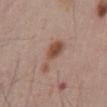Assessment:
Captured during whole-body skin photography for melanoma surveillance; the lesion was not biopsied.
Acquisition and patient details:
Automated image analysis of the tile measured a lesion-detection confidence of about 100/100. The recorded lesion diameter is about 5 mm. Captured under white-light illumination. The patient is a male aged 53–57. Cropped from a whole-body photographic skin survey; the tile spans about 15 mm. From the abdomen.